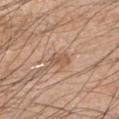<lesion>
  <biopsy_status>not biopsied; imaged during a skin examination</biopsy_status>
  <automated_metrics>
    <area_mm2_approx>3.5</area_mm2_approx>
    <eccentricity>0.75</eccentricity>
    <shape_asymmetry>0.35</shape_asymmetry>
    <border_irregularity_0_10>3.0</border_irregularity_0_10>
    <color_variation_0_10>3.5</color_variation_0_10>
    <peripheral_color_asymmetry>1.0</peripheral_color_asymmetry>
  </automated_metrics>
  <lesion_size>
    <long_diameter_mm_approx>2.5</long_diameter_mm_approx>
  </lesion_size>
  <image>
    <source>total-body photography crop</source>
    <field_of_view_mm>15</field_of_view_mm>
  </image>
  <site>left upper arm</site>
  <lighting>white-light</lighting>
  <patient>
    <sex>male</sex>
    <age_approx>50</age_approx>
  </patient>
</lesion>